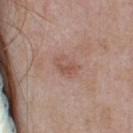No biopsy was performed on this lesion — it was imaged during a full skin examination and was not determined to be concerning. The tile uses white-light illumination. A female subject, aged around 30. The lesion is located on the head or neck. A 15 mm crop from a total-body photograph taken for skin-cancer surveillance.